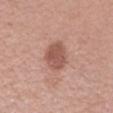Recorded during total-body skin imaging; not selected for excision or biopsy. The patient is a female about 50 years old. A roughly 15 mm field-of-view crop from a total-body skin photograph. The recorded lesion diameter is about 3.5 mm. This is a white-light tile. The lesion is located on the right forearm.